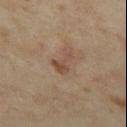Clinical impression: Captured during whole-body skin photography for melanoma surveillance; the lesion was not biopsied. Background: A female patient, approximately 35 years of age. Approximately 3 mm at its widest. Imaged with cross-polarized lighting. From the left thigh. A region of skin cropped from a whole-body photographic capture, roughly 15 mm wide.